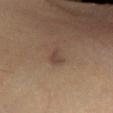<record>
  <biopsy_status>not biopsied; imaged during a skin examination</biopsy_status>
  <lesion_size>
    <long_diameter_mm_approx>2.5</long_diameter_mm_approx>
  </lesion_size>
  <lighting>cross-polarized</lighting>
  <site>left lower leg</site>
  <image>
    <source>total-body photography crop</source>
    <field_of_view_mm>15</field_of_view_mm>
  </image>
  <patient>
    <sex>male</sex>
    <age_approx>85+</age_approx>
  </patient>
</record>